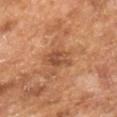workup: imaged on a skin check; not biopsied | diameter: ≈3.5 mm | illumination: cross-polarized | patient: male, in their mid- to late 60s | image: 15 mm crop, total-body photography.